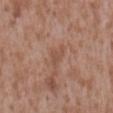notes = no biopsy performed (imaged during a skin exam) | automated lesion analysis = a normalized border contrast of about 5; an automated nevus-likeness rating near 0 out of 100 and a detector confidence of about 100 out of 100 that the crop contains a lesion | body site = the back | patient = male, aged 43 to 47 | tile lighting = white-light | lesion diameter = about 2.5 mm | image = total-body-photography crop, ~15 mm field of view.